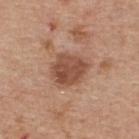The lesion was photographed on a routine skin check and not biopsied; there is no pathology result. Approximately 4 mm at its widest. This image is a 15 mm lesion crop taken from a total-body photograph. Located on the upper back. Imaged with white-light lighting. The subject is a male aged 63–67.